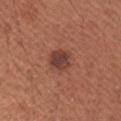Case summary:
- workup: total-body-photography surveillance lesion; no biopsy
- illumination: white-light
- acquisition: ~15 mm tile from a whole-body skin photo
- anatomic site: the right forearm
- subject: female, about 65 years old
- diameter: ≈3 mm